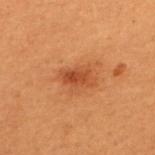Clinical impression: This lesion was catalogued during total-body skin photography and was not selected for biopsy. Clinical summary: A roughly 15 mm field-of-view crop from a total-body skin photograph. The lesion is located on the upper back. A male subject in their 50s. Automated image analysis of the tile measured a footprint of about 6 mm² and an eccentricity of roughly 0.85. And it measured a peripheral color-asymmetry measure near 1. Imaged with cross-polarized lighting.